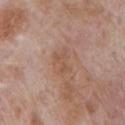{"biopsy_status": "not biopsied; imaged during a skin examination", "lesion_size": {"long_diameter_mm_approx": 3.0}, "lighting": "white-light", "site": "chest", "image": {"source": "total-body photography crop", "field_of_view_mm": 15}, "patient": {"sex": "male", "age_approx": 70}}